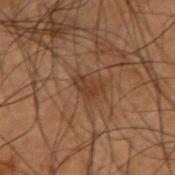Background:
The subject is a male in their 50s. From the right forearm. A 15 mm close-up tile from a total-body photography series done for melanoma screening.